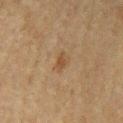Q: Was this lesion biopsied?
A: catalogued during a skin exam; not biopsied
Q: What lighting was used for the tile?
A: cross-polarized illumination
Q: What did automated image analysis measure?
A: a shape eccentricity near 0.85 and a symmetry-axis asymmetry near 0.3; a classifier nevus-likeness of about 15/100 and a lesion-detection confidence of about 100/100
Q: Where on the body is the lesion?
A: the mid back
Q: Lesion size?
A: ≈3 mm
Q: What are the patient's age and sex?
A: male, aged 73–77
Q: What kind of image is this?
A: total-body-photography crop, ~15 mm field of view